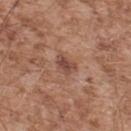biopsy_status: not biopsied; imaged during a skin examination
site: upper back
patient:
  sex: male
  age_approx: 55
image:
  source: total-body photography crop
  field_of_view_mm: 15